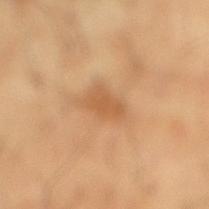No biopsy was performed on this lesion — it was imaged during a full skin examination and was not determined to be concerning.
The lesion is on the right lower leg.
A male patient aged approximately 65.
A 15 mm crop from a total-body photograph taken for skin-cancer surveillance.